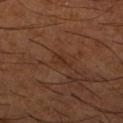The lesion was photographed on a routine skin check and not biopsied; there is no pathology result.
This is a cross-polarized tile.
A roughly 15 mm field-of-view crop from a total-body skin photograph.
A male subject, about 65 years old.
The lesion is on the right lower leg.
An algorithmic analysis of the crop reported a mean CIELAB color near L≈30 a*≈20 b*≈28, about 5 CIELAB-L* units darker than the surrounding skin, and a lesion-to-skin contrast of about 5.5 (normalized; higher = more distinct). And it measured a border-irregularity index near 3.5/10 and internal color variation of about 1 on a 0–10 scale. And it measured a lesion-detection confidence of about 85/100.